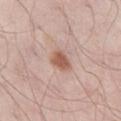Part of a total-body skin-imaging series; this lesion was reviewed on a skin check and was not flagged for biopsy. The lesion is located on the left thigh. A male patient, aged 53 to 57. Imaged with white-light lighting. A region of skin cropped from a whole-body photographic capture, roughly 15 mm wide.